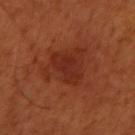workup: no biopsy performed (imaged during a skin exam)
image-analysis metrics: a footprint of about 9 mm², an eccentricity of roughly 0.8, and a shape-asymmetry score of about 0.35 (0 = symmetric); a lesion color around L≈30 a*≈29 b*≈31 in CIELAB, a lesion–skin lightness drop of about 7, and a normalized border contrast of about 6.5; a detector confidence of about 100 out of 100 that the crop contains a lesion
subject: male, approximately 50 years of age
tile lighting: cross-polarized
site: the right upper arm
image source: ~15 mm crop, total-body skin-cancer survey